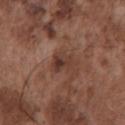Captured during whole-body skin photography for melanoma surveillance; the lesion was not biopsied.
This is a white-light tile.
The lesion is on the chest.
A male subject, roughly 75 years of age.
A close-up tile cropped from a whole-body skin photograph, about 15 mm across.